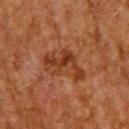Impression:
Recorded during total-body skin imaging; not selected for excision or biopsy.
Acquisition and patient details:
The patient is a male about 60 years old. The tile uses cross-polarized illumination. From the upper back. Automated image analysis of the tile measured a shape eccentricity near 0.85 and a symmetry-axis asymmetry near 0.65. And it measured a mean CIELAB color near L≈29 a*≈21 b*≈28. A roughly 15 mm field-of-view crop from a total-body skin photograph. Measured at roughly 5.5 mm in maximum diameter.